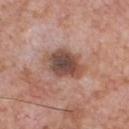{
  "biopsy_status": "not biopsied; imaged during a skin examination",
  "site": "chest",
  "lighting": "white-light",
  "image": {
    "source": "total-body photography crop",
    "field_of_view_mm": 15
  },
  "patient": {
    "sex": "male",
    "age_approx": 60
  },
  "lesion_size": {
    "long_diameter_mm_approx": 5.5
  },
  "automated_metrics": {
    "area_mm2_approx": 14.0,
    "eccentricity": 0.75,
    "shape_asymmetry": 0.2,
    "cielab_L": 49,
    "cielab_a": 20,
    "cielab_b": 25,
    "vs_skin_darker_L": 14.0,
    "vs_skin_contrast_norm": 9.5
  }
}